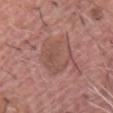The lesion was tiled from a total-body skin photograph and was not biopsied. Approximately 4.5 mm at its widest. A male patient aged 78 to 82. The tile uses white-light illumination. Cropped from a total-body skin-imaging series; the visible field is about 15 mm. The lesion is located on the chest.